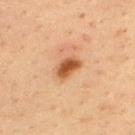{
  "biopsy_status": "not biopsied; imaged during a skin examination",
  "lighting": "cross-polarized",
  "lesion_size": {
    "long_diameter_mm_approx": 3.5
  },
  "automated_metrics": {
    "area_mm2_approx": 5.5,
    "eccentricity": 0.8,
    "shape_asymmetry": 0.25
  },
  "patient": {
    "sex": "male",
    "age_approx": 35
  },
  "site": "back",
  "image": {
    "source": "total-body photography crop",
    "field_of_view_mm": 15
  }
}